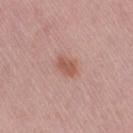  biopsy_status: not biopsied; imaged during a skin examination
  site: leg
  automated_metrics:
    area_mm2_approx: 4.0
    eccentricity: 0.7
    cielab_L: 55
    cielab_a: 23
    cielab_b: 28
    vs_skin_darker_L: 9.0
    nevus_likeness_0_100: 80
  image:
    source: total-body photography crop
    field_of_view_mm: 15
  patient:
    sex: female
    age_approx: 50